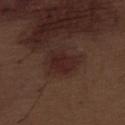workup = total-body-photography surveillance lesion; no biopsy
lesion size = ~4 mm (longest diameter)
site = the abdomen
image source = total-body-photography crop, ~15 mm field of view
subject = male, aged approximately 70